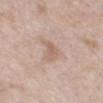  biopsy_status: not biopsied; imaged during a skin examination
  image:
    source: total-body photography crop
    field_of_view_mm: 15
  site: back
  patient:
    sex: male
    age_approx: 60